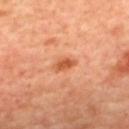notes: total-body-photography surveillance lesion; no biopsy | automated metrics: a footprint of about 3.5 mm², an outline eccentricity of about 0.85 (0 = round, 1 = elongated), and a shape-asymmetry score of about 0.25 (0 = symmetric); a lesion color around L≈55 a*≈30 b*≈40 in CIELAB, a lesion–skin lightness drop of about 11, and a lesion-to-skin contrast of about 7.5 (normalized; higher = more distinct); border irregularity of about 2.5 on a 0–10 scale, a color-variation rating of about 2/10, and radial color variation of about 1; an automated nevus-likeness rating near 70 out of 100 | illumination: cross-polarized illumination | acquisition: total-body-photography crop, ~15 mm field of view | lesion diameter: about 3 mm | subject: female, in their mid- to late 40s | site: the back.